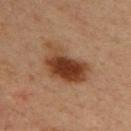The lesion was tiled from a total-body skin photograph and was not biopsied.
Captured under cross-polarized illumination.
The lesion is on the upper back.
A close-up tile cropped from a whole-body skin photograph, about 15 mm across.
The subject is a male roughly 35 years of age.
Approximately 6 mm at its widest.